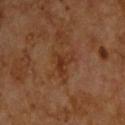biopsy_status: not biopsied; imaged during a skin examination
patient:
  sex: male
  age_approx: 60
lesion_size:
  long_diameter_mm_approx: 3.0
lighting: cross-polarized
site: upper back
automated_metrics:
  cielab_L: 30
  cielab_a: 20
  cielab_b: 29
  vs_skin_contrast_norm: 6.5
image:
  source: total-body photography crop
  field_of_view_mm: 15